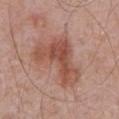{
  "biopsy_status": "not biopsied; imaged during a skin examination",
  "automated_metrics": {
    "cielab_L": 51,
    "cielab_a": 24,
    "cielab_b": 28,
    "vs_skin_darker_L": 10.0,
    "vs_skin_contrast_norm": 7.5,
    "border_irregularity_0_10": 7.0,
    "color_variation_0_10": 4.5,
    "nevus_likeness_0_100": 10,
    "lesion_detection_confidence_0_100": 100
  },
  "patient": {
    "sex": "male",
    "age_approx": 70
  },
  "site": "chest",
  "lighting": "white-light",
  "image": {
    "source": "total-body photography crop",
    "field_of_view_mm": 15
  }
}